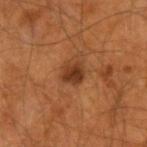Context:
The lesion-visualizer software estimated an area of roughly 5 mm² and two-axis asymmetry of about 0.2. The software also gave a lesion color around L≈33 a*≈23 b*≈32 in CIELAB, a lesion–skin lightness drop of about 11, and a lesion-to-skin contrast of about 9.5 (normalized; higher = more distinct). About 2.5 mm across. Captured under cross-polarized illumination. The patient is a male about 55 years old. Located on the right forearm. Cropped from a whole-body photographic skin survey; the tile spans about 15 mm.